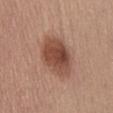{
  "biopsy_status": "not biopsied; imaged during a skin examination",
  "site": "abdomen",
  "image": {
    "source": "total-body photography crop",
    "field_of_view_mm": 15
  },
  "patient": {
    "sex": "male",
    "age_approx": 55
  },
  "automated_metrics": {
    "area_mm2_approx": 17.0,
    "shape_asymmetry": 0.15,
    "cielab_L": 48,
    "cielab_a": 22,
    "cielab_b": 28,
    "vs_skin_darker_L": 13.0,
    "vs_skin_contrast_norm": 9.0
  },
  "lighting": "white-light"
}